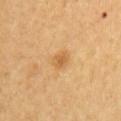  biopsy_status: not biopsied; imaged during a skin examination